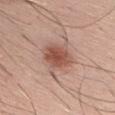The lesion was tiled from a total-body skin photograph and was not biopsied.
Imaged with white-light lighting.
A male subject roughly 45 years of age.
Cropped from a total-body skin-imaging series; the visible field is about 15 mm.
About 4 mm across.
On the chest.